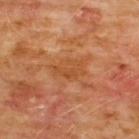diameter — ~4 mm (longest diameter)
illumination — cross-polarized
imaging modality — total-body-photography crop, ~15 mm field of view
anatomic site — the chest
patient — male, approximately 60 years of age